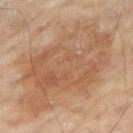Q: Was this lesion biopsied?
A: total-body-photography surveillance lesion; no biopsy
Q: Automated lesion metrics?
A: an area of roughly 65 mm² and an outline eccentricity of about 0.85 (0 = round, 1 = elongated); a lesion color around L≈57 a*≈20 b*≈33 in CIELAB and a lesion-to-skin contrast of about 6 (normalized; higher = more distinct); a color-variation rating of about 5/10; a nevus-likeness score of about 40/100
Q: What is the lesion's diameter?
A: about 13 mm
Q: Lesion location?
A: the right thigh
Q: How was this image acquired?
A: total-body-photography crop, ~15 mm field of view
Q: How was the tile lit?
A: cross-polarized illumination
Q: What are the patient's age and sex?
A: male, roughly 65 years of age